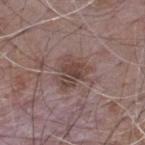Q: Was a biopsy performed?
A: imaged on a skin check; not biopsied
Q: What are the patient's age and sex?
A: male, in their mid- to late 60s
Q: What did automated image analysis measure?
A: an average lesion color of about L≈44 a*≈16 b*≈20 (CIELAB), about 9 CIELAB-L* units darker than the surrounding skin, and a normalized lesion–skin contrast near 7.5
Q: How was the tile lit?
A: white-light
Q: Lesion size?
A: about 4.5 mm
Q: Lesion location?
A: the back
Q: What is the imaging modality?
A: total-body-photography crop, ~15 mm field of view